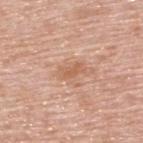• follow-up: imaged on a skin check; not biopsied
• patient: male, aged 78–82
• image-analysis metrics: a footprint of about 3.5 mm², an eccentricity of roughly 0.9, and two-axis asymmetry of about 0.3; a border-irregularity rating of about 3/10 and a within-lesion color-variation index near 1/10
• illumination: white-light illumination
• site: the upper back
• acquisition: ~15 mm tile from a whole-body skin photo
• lesion diameter: ~3 mm (longest diameter)